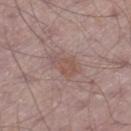This lesion was catalogued during total-body skin photography and was not selected for biopsy. Imaged with white-light lighting. Automated image analysis of the tile measured a lesion color around L≈52 a*≈18 b*≈22 in CIELAB and a normalized border contrast of about 5.5. It also reported border irregularity of about 3.5 on a 0–10 scale, a color-variation rating of about 2/10, and peripheral color asymmetry of about 0.5. The analysis additionally found a nevus-likeness score of about 0/100 and a detector confidence of about 100 out of 100 that the crop contains a lesion. Located on the left thigh. A 15 mm crop from a total-body photograph taken for skin-cancer surveillance. A male patient aged 63 to 67. Measured at roughly 3.5 mm in maximum diameter.